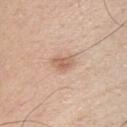Q: Is there a histopathology result?
A: no biopsy performed (imaged during a skin exam)
Q: What are the patient's age and sex?
A: male, roughly 30 years of age
Q: What is the anatomic site?
A: the chest
Q: What kind of image is this?
A: 15 mm crop, total-body photography
Q: Illumination type?
A: white-light
Q: What did automated image analysis measure?
A: an area of roughly 4.5 mm² and a shape-asymmetry score of about 0.35 (0 = symmetric)